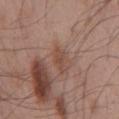biopsy status=imaged on a skin check; not biopsied | illumination=white-light illumination | diameter=~2.5 mm (longest diameter) | body site=the chest | patient=male, aged 53 to 57 | imaging modality=total-body-photography crop, ~15 mm field of view.